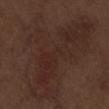Q: Was a biopsy performed?
A: no biopsy performed (imaged during a skin exam)